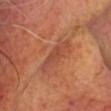follow-up=total-body-photography surveillance lesion; no biopsy | body site=the head or neck | patient=roughly 65 years of age | image source=~15 mm tile from a whole-body skin photo | tile lighting=cross-polarized | automated lesion analysis=a mean CIELAB color near L≈47 a*≈28 b*≈32 and a normalized border contrast of about 6; a nevus-likeness score of about 0/100 and lesion-presence confidence of about 95/100.